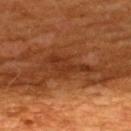Assessment:
This lesion was catalogued during total-body skin photography and was not selected for biopsy.
Clinical summary:
A male subject, aged around 60. A 15 mm close-up extracted from a 3D total-body photography capture. The lesion is on the upper back.